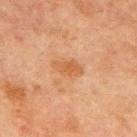Assessment: This lesion was catalogued during total-body skin photography and was not selected for biopsy. Acquisition and patient details: Automated tile analysis of the lesion measured a lesion color around L≈47 a*≈21 b*≈34 in CIELAB, about 7 CIELAB-L* units darker than the surrounding skin, and a lesion-to-skin contrast of about 6.5 (normalized; higher = more distinct). And it measured a nevus-likeness score of about 5/100 and a detector confidence of about 100 out of 100 that the crop contains a lesion. The recorded lesion diameter is about 3 mm. The subject is a male aged around 65. On the front of the torso. A 15 mm close-up tile from a total-body photography series done for melanoma screening.